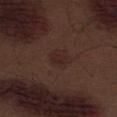Impression:
Recorded during total-body skin imaging; not selected for excision or biopsy.
Background:
On the abdomen. The lesion-visualizer software estimated a classifier nevus-likeness of about 85/100 and a detector confidence of about 100 out of 100 that the crop contains a lesion. Cropped from a total-body skin-imaging series; the visible field is about 15 mm. The recorded lesion diameter is about 2.5 mm. The patient is a male aged 68–72.